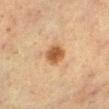biopsy_status: not biopsied; imaged during a skin examination
patient:
  sex: female
  age_approx: 55
image:
  source: total-body photography crop
  field_of_view_mm: 15
automated_metrics:
  area_mm2_approx: 6.0
  eccentricity: 0.6
  nevus_likeness_0_100: 100
site: left lower leg